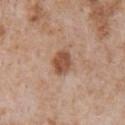{"biopsy_status": "not biopsied; imaged during a skin examination", "site": "chest", "lighting": "white-light", "patient": {"sex": "male", "age_approx": 65}, "image": {"source": "total-body photography crop", "field_of_view_mm": 15}}